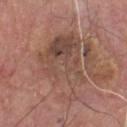Image and clinical context:
The total-body-photography lesion software estimated an average lesion color of about L≈48 a*≈19 b*≈25 (CIELAB) and a normalized border contrast of about 6. It also reported a border-irregularity rating of about 6/10, a within-lesion color-variation index near 6.5/10, and peripheral color asymmetry of about 2.5. The analysis additionally found a classifier nevus-likeness of about 0/100 and a lesion-detection confidence of about 95/100. Measured at roughly 8 mm in maximum diameter. A 15 mm crop from a total-body photograph taken for skin-cancer surveillance. A male subject, in their mid-70s. The lesion is located on the chest.